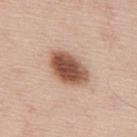No biopsy was performed on this lesion — it was imaged during a full skin examination and was not determined to be concerning.
Approximately 5 mm at its widest.
A male subject, aged around 45.
The lesion is located on the mid back.
A region of skin cropped from a whole-body photographic capture, roughly 15 mm wide.